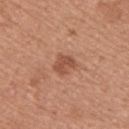• notes · catalogued during a skin exam; not biopsied
• acquisition · total-body-photography crop, ~15 mm field of view
• site · the upper back
• lighting · white-light
• patient · female, aged 33 to 37